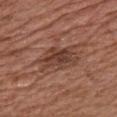No biopsy was performed on this lesion — it was imaged during a full skin examination and was not determined to be concerning.
The recorded lesion diameter is about 5.5 mm.
The lesion is located on the chest.
A male subject, in their mid-60s.
A 15 mm close-up tile from a total-body photography series done for melanoma screening.
This is a white-light tile.